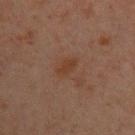Assessment:
Recorded during total-body skin imaging; not selected for excision or biopsy.
Acquisition and patient details:
Automated tile analysis of the lesion measured a mean CIELAB color near L≈31 a*≈17 b*≈25 and roughly 5 lightness units darker than nearby skin. And it measured a border-irregularity rating of about 3/10, a within-lesion color-variation index near 1.5/10, and a peripheral color-asymmetry measure near 0.5. The analysis additionally found a detector confidence of about 100 out of 100 that the crop contains a lesion. Imaged with cross-polarized lighting. A male patient, aged 28 to 32. The lesion's longest dimension is about 2.5 mm. A 15 mm close-up tile from a total-body photography series done for melanoma screening. From the upper back.